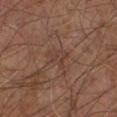Case summary:
* biopsy status · imaged on a skin check; not biopsied
* lesion size · about 3 mm
* acquisition · ~15 mm crop, total-body skin-cancer survey
* lighting · cross-polarized
* subject · male, aged 58 to 62
* site · the right leg